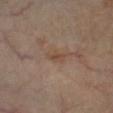follow-up: total-body-photography surveillance lesion; no biopsy | image: total-body-photography crop, ~15 mm field of view | body site: the left lower leg | lesion diameter: ≈2.5 mm | illumination: cross-polarized illumination | image-analysis metrics: a lesion area of about 3 mm² and a shape-asymmetry score of about 0.4 (0 = symmetric); a lesion color around L≈44 a*≈16 b*≈26 in CIELAB, about 6 CIELAB-L* units darker than the surrounding skin, and a normalized lesion–skin contrast near 5.5; a border-irregularity index near 4/10 and radial color variation of about 0; an automated nevus-likeness rating near 0 out of 100 | patient: male, in their 60s.